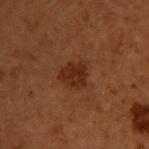Q: Was a biopsy performed?
A: imaged on a skin check; not biopsied
Q: What are the patient's age and sex?
A: male, aged 48 to 52
Q: What did automated image analysis measure?
A: a lesion area of about 7 mm², an eccentricity of roughly 0.65, and a shape-asymmetry score of about 0.25 (0 = symmetric); an average lesion color of about L≈21 a*≈19 b*≈25 (CIELAB), about 7 CIELAB-L* units darker than the surrounding skin, and a normalized border contrast of about 8; a within-lesion color-variation index near 1.5/10 and a peripheral color-asymmetry measure near 0.5
Q: How was the tile lit?
A: cross-polarized illumination
Q: What is the lesion's diameter?
A: ≈3.5 mm
Q: What kind of image is this?
A: ~15 mm crop, total-body skin-cancer survey
Q: Lesion location?
A: the upper back